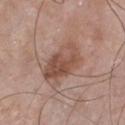biopsy status: imaged on a skin check; not biopsied | tile lighting: white-light | acquisition: ~15 mm tile from a whole-body skin photo | automated metrics: a footprint of about 14 mm², an eccentricity of roughly 0.85, and a shape-asymmetry score of about 0.25 (0 = symmetric); a within-lesion color-variation index near 6/10; an automated nevus-likeness rating near 10 out of 100 and a detector confidence of about 100 out of 100 that the crop contains a lesion | subject: male, aged 53 to 57 | location: the front of the torso | size: ~5.5 mm (longest diameter).